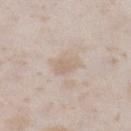Notes:
- biopsy status · no biopsy performed (imaged during a skin exam)
- lesion diameter · ≈3 mm
- subject · female, approximately 25 years of age
- illumination · white-light
- image · ~15 mm crop, total-body skin-cancer survey
- TBP lesion metrics · roughly 6 lightness units darker than nearby skin and a normalized lesion–skin contrast near 4.5; a border-irregularity index near 2.5/10, a within-lesion color-variation index near 1.5/10, and peripheral color asymmetry of about 0.5
- anatomic site · the left lower leg